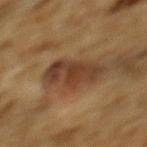Q: Was this lesion biopsied?
A: no biopsy performed (imaged during a skin exam)
Q: Lesion location?
A: the mid back
Q: Patient demographics?
A: male, aged around 85 or older
Q: What lighting was used for the tile?
A: cross-polarized
Q: What is the lesion's diameter?
A: ≈5.5 mm
Q: How was this image acquired?
A: total-body-photography crop, ~15 mm field of view